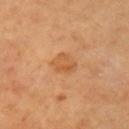notes: imaged on a skin check; not biopsied | site: the arm | subject: female, aged approximately 60 | diameter: about 3 mm | imaging modality: total-body-photography crop, ~15 mm field of view.